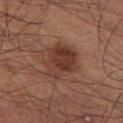- biopsy status · no biopsy performed (imaged during a skin exam)
- acquisition · ~15 mm crop, total-body skin-cancer survey
- anatomic site · the left thigh
- lesion diameter · about 5 mm
- subject · male, in their 50s
- lighting · cross-polarized